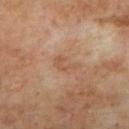<tbp_lesion>
  <biopsy_status>not biopsied; imaged during a skin examination</biopsy_status>
  <lesion_size>
    <long_diameter_mm_approx>3.0</long_diameter_mm_approx>
  </lesion_size>
  <image>
    <source>total-body photography crop</source>
    <field_of_view_mm>15</field_of_view_mm>
  </image>
  <site>mid back</site>
  <lighting>cross-polarized</lighting>
  <patient>
    <sex>male</sex>
    <age_approx>70</age_approx>
  </patient>
</tbp_lesion>